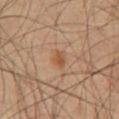<lesion>
  <biopsy_status>not biopsied; imaged during a skin examination</biopsy_status>
  <lighting>cross-polarized</lighting>
  <site>chest</site>
  <lesion_size>
    <long_diameter_mm_approx>2.5</long_diameter_mm_approx>
  </lesion_size>
  <automated_metrics>
    <area_mm2_approx>3.5</area_mm2_approx>
    <shape_asymmetry>0.25</shape_asymmetry>
    <border_irregularity_0_10>2.0</border_irregularity_0_10>
    <color_variation_0_10>2.5</color_variation_0_10>
    <peripheral_color_asymmetry>1.0</peripheral_color_asymmetry>
    <lesion_detection_confidence_0_100>100</lesion_detection_confidence_0_100>
  </automated_metrics>
  <patient>
    <sex>male</sex>
    <age_approx>55</age_approx>
  </patient>
  <image>
    <source>total-body photography crop</source>
    <field_of_view_mm>15</field_of_view_mm>
  </image>
</lesion>